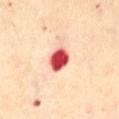Case summary:
- biopsy status: total-body-photography surveillance lesion; no biopsy
- tile lighting: cross-polarized
- subject: female, in their 60s
- location: the abdomen
- size: about 3 mm
- imaging modality: 15 mm crop, total-body photography